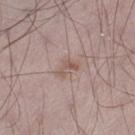Clinical impression:
The lesion was tiled from a total-body skin photograph and was not biopsied.
Clinical summary:
Imaged with white-light lighting. A 15 mm crop from a total-body photograph taken for skin-cancer surveillance. An algorithmic analysis of the crop reported an average lesion color of about L≈55 a*≈17 b*≈23 (CIELAB), a lesion–skin lightness drop of about 7, and a normalized border contrast of about 6. A male subject roughly 70 years of age. Located on the leg. The recorded lesion diameter is about 3 mm.